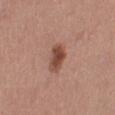biopsy status: catalogued during a skin exam; not biopsied
image: ~15 mm tile from a whole-body skin photo
patient: female, aged 33–37
anatomic site: the left lower leg